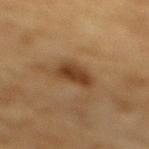No biopsy was performed on this lesion — it was imaged during a full skin examination and was not determined to be concerning. On the mid back. The patient is a male aged 83 or older. The tile uses cross-polarized illumination. A 15 mm crop from a total-body photograph taken for skin-cancer surveillance.